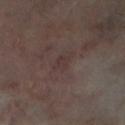{
  "biopsy_status": "not biopsied; imaged during a skin examination",
  "site": "left lower leg",
  "patient": {
    "sex": "female",
    "age_approx": 60
  },
  "lighting": "cross-polarized",
  "lesion_size": {
    "long_diameter_mm_approx": 3.0
  },
  "image": {
    "source": "total-body photography crop",
    "field_of_view_mm": 15
  }
}